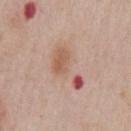Clinical impression:
This lesion was catalogued during total-body skin photography and was not selected for biopsy.
Image and clinical context:
The subject is a male approximately 60 years of age. The lesion is on the chest. Automated image analysis of the tile measured an area of roughly 13 mm², an eccentricity of roughly 0.85, and a symmetry-axis asymmetry near 0.5. The software also gave roughly 9 lightness units darker than nearby skin and a normalized lesion–skin contrast near 6. The software also gave an automated nevus-likeness rating near 0 out of 100. A 15 mm crop from a total-body photograph taken for skin-cancer surveillance. The lesion's longest dimension is about 6 mm. Captured under white-light illumination.